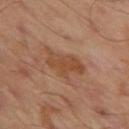biopsy_status: not biopsied; imaged during a skin examination
site: left thigh
automated_metrics:
  border_irregularity_0_10: 4.5
  color_variation_0_10: 3.0
  peripheral_color_asymmetry: 1.0
  nevus_likeness_0_100: 0
  lesion_detection_confidence_0_100: 100
image:
  source: total-body photography crop
  field_of_view_mm: 15
patient:
  sex: male
  age_approx: 45
lesion_size:
  long_diameter_mm_approx: 5.0